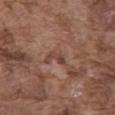No biopsy was performed on this lesion — it was imaged during a full skin examination and was not determined to be concerning.
Located on the abdomen.
Measured at roughly 2.5 mm in maximum diameter.
A male subject, aged approximately 75.
A close-up tile cropped from a whole-body skin photograph, about 15 mm across.
The total-body-photography lesion software estimated a color-variation rating of about 0/10 and a peripheral color-asymmetry measure near 0.
This is a white-light tile.